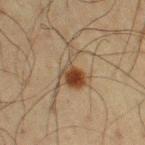This lesion was catalogued during total-body skin photography and was not selected for biopsy.
The lesion is on the right upper arm.
The patient is a male aged around 60.
Cropped from a total-body skin-imaging series; the visible field is about 15 mm.
The recorded lesion diameter is about 5.5 mm.
The tile uses cross-polarized illumination.